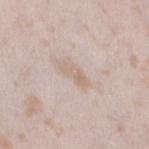No biopsy was performed on this lesion — it was imaged during a full skin examination and was not determined to be concerning.
A 15 mm close-up tile from a total-body photography series done for melanoma screening.
A female patient roughly 25 years of age.
Longest diameter approximately 4 mm.
On the left thigh.